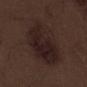Captured during whole-body skin photography for melanoma surveillance; the lesion was not biopsied. Located on the leg. The tile uses white-light illumination. Automated tile analysis of the lesion measured a classifier nevus-likeness of about 75/100 and a lesion-detection confidence of about 100/100. The recorded lesion diameter is about 8.5 mm. A roughly 15 mm field-of-view crop from a total-body skin photograph. A male subject approximately 70 years of age.